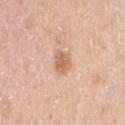Q: Is there a histopathology result?
A: no biopsy performed (imaged during a skin exam)
Q: Who is the patient?
A: female, aged 48 to 52
Q: What is the anatomic site?
A: the right upper arm
Q: How was this image acquired?
A: ~15 mm tile from a whole-body skin photo
Q: How large is the lesion?
A: ≈2.5 mm
Q: How was the tile lit?
A: white-light